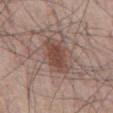Recorded during total-body skin imaging; not selected for excision or biopsy.
A 15 mm close-up tile from a total-body photography series done for melanoma screening.
The tile uses white-light illumination.
Longest diameter approximately 4.5 mm.
A male patient aged approximately 65.
The lesion is on the front of the torso.